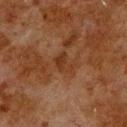This lesion was catalogued during total-body skin photography and was not selected for biopsy. A male patient about 80 years old. A lesion tile, about 15 mm wide, cut from a 3D total-body photograph. Longest diameter approximately 3 mm. An algorithmic analysis of the crop reported a border-irregularity index near 4/10, internal color variation of about 2 on a 0–10 scale, and radial color variation of about 0.5. And it measured a nevus-likeness score of about 0/100 and lesion-presence confidence of about 100/100. The tile uses cross-polarized illumination. Located on the upper back.